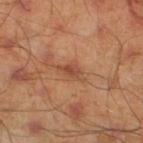Cropped from a total-body skin-imaging series; the visible field is about 15 mm.
The recorded lesion diameter is about 3 mm.
The lesion is on the leg.
Imaged with cross-polarized lighting.
A male patient approximately 45 years of age.